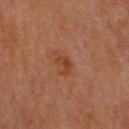Assessment:
Imaged during a routine full-body skin examination; the lesion was not biopsied and no histopathology is available.
Clinical summary:
A 15 mm crop from a total-body photograph taken for skin-cancer surveillance. The lesion is on the upper back. The patient is a male aged approximately 70. The lesion's longest dimension is about 2.5 mm.